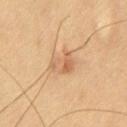Q: Is there a histopathology result?
A: catalogued during a skin exam; not biopsied
Q: What is the anatomic site?
A: the chest
Q: What is the imaging modality?
A: ~15 mm crop, total-body skin-cancer survey
Q: Who is the patient?
A: male, about 70 years old
Q: How large is the lesion?
A: ≈3.5 mm